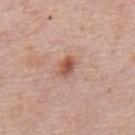Impression:
No biopsy was performed on this lesion — it was imaged during a full skin examination and was not determined to be concerning.
Background:
Approximately 2.5 mm at its widest. Imaged with white-light lighting. A region of skin cropped from a whole-body photographic capture, roughly 15 mm wide. The lesion-visualizer software estimated a footprint of about 4 mm² and an eccentricity of roughly 0.7. It also reported an average lesion color of about L≈54 a*≈23 b*≈29 (CIELAB), roughly 12 lightness units darker than nearby skin, and a normalized border contrast of about 8.5. And it measured a border-irregularity rating of about 2/10, a color-variation rating of about 3.5/10, and peripheral color asymmetry of about 1.5. It also reported a nevus-likeness score of about 90/100 and lesion-presence confidence of about 100/100. The lesion is located on the back. The subject is a male in their mid- to late 70s.